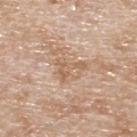Assessment:
Captured during whole-body skin photography for melanoma surveillance; the lesion was not biopsied.
Context:
The subject is a male aged around 80. A 15 mm close-up tile from a total-body photography series done for melanoma screening. Measured at roughly 3.5 mm in maximum diameter. Located on the upper back. Automated tile analysis of the lesion measured a footprint of about 4 mm² and an eccentricity of roughly 0.85. This is a white-light tile.